A male subject, aged 63–67. A lesion tile, about 15 mm wide, cut from a 3D total-body photograph. From the mid back:
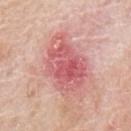Acquisition and patient details:
The lesion's longest dimension is about 6.5 mm. The tile uses white-light illumination.
Diagnosis:
Histopathology of the biopsied lesion showed a superficial basal cell carcinoma — a skin cancer.